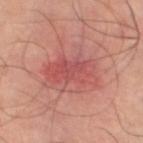Acquisition and patient details: The lesion's longest dimension is about 5 mm. A 15 mm crop from a total-body photograph taken for skin-cancer surveillance. The tile uses cross-polarized illumination. The lesion is on the left thigh.